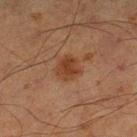Captured during whole-body skin photography for melanoma surveillance; the lesion was not biopsied. A male subject, aged 63–67. An algorithmic analysis of the crop reported a lesion color around L≈32 a*≈19 b*≈27 in CIELAB, roughly 8 lightness units darker than nearby skin, and a normalized border contrast of about 8. And it measured a border-irregularity rating of about 1.5/10, a within-lesion color-variation index near 2/10, and peripheral color asymmetry of about 1. The analysis additionally found a lesion-detection confidence of about 100/100. The lesion is on the right lower leg. A 15 mm crop from a total-body photograph taken for skin-cancer surveillance. About 3 mm across. The tile uses cross-polarized illumination.